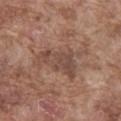| feature | finding |
|---|---|
| follow-up | catalogued during a skin exam; not biopsied |
| lighting | white-light illumination |
| acquisition | ~15 mm crop, total-body skin-cancer survey |
| anatomic site | the abdomen |
| diameter | ~7 mm (longest diameter) |
| patient | male, aged around 75 |
| automated lesion analysis | a lesion area of about 17 mm²; a mean CIELAB color near L≈48 a*≈18 b*≈25, a lesion–skin lightness drop of about 8, and a lesion-to-skin contrast of about 6 (normalized; higher = more distinct); a border-irregularity rating of about 8.5/10, a color-variation rating of about 3/10, and radial color variation of about 1 |